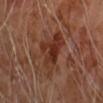Findings:
* lighting — cross-polarized
* lesion size — about 5 mm
* location — the right forearm
* automated lesion analysis — a lesion area of about 13 mm², an eccentricity of roughly 0.7, and a symmetry-axis asymmetry near 0.45; a lesion color around L≈32 a*≈23 b*≈27 in CIELAB; a classifier nevus-likeness of about 5/100 and a lesion-detection confidence of about 100/100
* patient — male, aged around 70
* image source — 15 mm crop, total-body photography